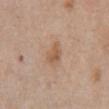Impression:
Captured during whole-body skin photography for melanoma surveillance; the lesion was not biopsied.
Acquisition and patient details:
The subject is a male in their 80s. From the abdomen. A region of skin cropped from a whole-body photographic capture, roughly 15 mm wide.